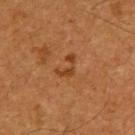The lesion was photographed on a routine skin check and not biopsied; there is no pathology result.
A male subject, aged approximately 65.
About 3 mm across.
On the upper back.
Automated image analysis of the tile measured a lesion area of about 3.5 mm², a shape eccentricity near 0.8, and a symmetry-axis asymmetry near 0.75. It also reported border irregularity of about 8.5 on a 0–10 scale, a within-lesion color-variation index near 0/10, and radial color variation of about 0. The analysis additionally found a classifier nevus-likeness of about 0/100 and lesion-presence confidence of about 100/100.
Cropped from a whole-body photographic skin survey; the tile spans about 15 mm.
Imaged with cross-polarized lighting.